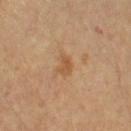Part of a total-body skin-imaging series; this lesion was reviewed on a skin check and was not flagged for biopsy.
From the left upper arm.
This is a cross-polarized tile.
A 15 mm close-up tile from a total-body photography series done for melanoma screening.
A male patient, aged approximately 55.
The recorded lesion diameter is about 2.5 mm.
The lesion-visualizer software estimated a detector confidence of about 100 out of 100 that the crop contains a lesion.